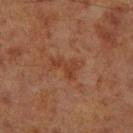  site: right lower leg
  image:
    source: total-body photography crop
    field_of_view_mm: 15
  lesion_size:
    long_diameter_mm_approx: 4.0
  patient:
    sex: male
    age_approx: 70
  lighting: cross-polarized